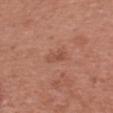Imaged during a routine full-body skin examination; the lesion was not biopsied and no histopathology is available.
The lesion's longest dimension is about 2.5 mm.
The tile uses white-light illumination.
A female patient about 40 years old.
A region of skin cropped from a whole-body photographic capture, roughly 15 mm wide.
From the right upper arm.
The total-body-photography lesion software estimated a lesion-detection confidence of about 100/100.